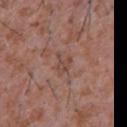Q: Was a biopsy performed?
A: imaged on a skin check; not biopsied
Q: What did automated image analysis measure?
A: a shape eccentricity near 0.7 and a symmetry-axis asymmetry near 0.4; a border-irregularity rating of about 4/10 and internal color variation of about 3.5 on a 0–10 scale; an automated nevus-likeness rating near 0 out of 100
Q: What is the imaging modality?
A: 15 mm crop, total-body photography
Q: Patient demographics?
A: male, aged around 45
Q: Illumination type?
A: white-light
Q: What is the anatomic site?
A: the chest